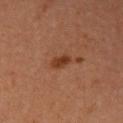Located on the right upper arm.
A female subject, roughly 35 years of age.
A 15 mm crop from a total-body photograph taken for skin-cancer surveillance.
Captured under cross-polarized illumination.
The recorded lesion diameter is about 2.5 mm.